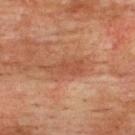Impression:
Captured during whole-body skin photography for melanoma surveillance; the lesion was not biopsied.
Context:
A male patient, aged 73–77. Imaged with cross-polarized lighting. On the upper back. The recorded lesion diameter is about 4.5 mm. A close-up tile cropped from a whole-body skin photograph, about 15 mm across. An algorithmic analysis of the crop reported an area of roughly 5.5 mm², an outline eccentricity of about 0.95 (0 = round, 1 = elongated), and two-axis asymmetry of about 0.45. And it measured an average lesion color of about L≈41 a*≈22 b*≈28 (CIELAB), roughly 7 lightness units darker than nearby skin, and a normalized border contrast of about 5.5. The analysis additionally found a border-irregularity index near 6/10 and radial color variation of about 0.5.